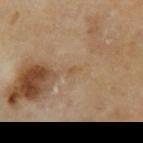Case summary:
- follow-up · no biopsy performed (imaged during a skin exam)
- illumination · cross-polarized
- patient · male, aged 68 to 72
- lesion diameter · about 1 mm
- image · ~15 mm crop, total-body skin-cancer survey
- body site · the arm